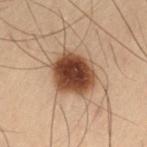This lesion was catalogued during total-body skin photography and was not selected for biopsy.
On the left thigh.
Cropped from a total-body skin-imaging series; the visible field is about 15 mm.
The subject is a male in their mid-50s.